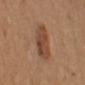Findings:
- follow-up — total-body-photography surveillance lesion; no biopsy
- anatomic site — the chest
- imaging modality — total-body-photography crop, ~15 mm field of view
- subject — female, aged around 40